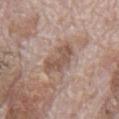Part of a total-body skin-imaging series; this lesion was reviewed on a skin check and was not flagged for biopsy. A male patient, in their 70s. On the mid back. A 15 mm crop from a total-body photograph taken for skin-cancer surveillance.